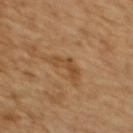Notes:
* biopsy status: catalogued during a skin exam; not biopsied
* illumination: cross-polarized illumination
* subject: female, roughly 55 years of age
* acquisition: ~15 mm crop, total-body skin-cancer survey
* location: the upper back